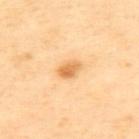Clinical impression: This lesion was catalogued during total-body skin photography and was not selected for biopsy. Acquisition and patient details: The total-body-photography lesion software estimated a border-irregularity rating of about 2/10, a color-variation rating of about 4/10, and a peripheral color-asymmetry measure near 1.5. Measured at roughly 3 mm in maximum diameter. From the upper back. A male subject, aged around 65. Imaged with cross-polarized lighting. A lesion tile, about 15 mm wide, cut from a 3D total-body photograph.